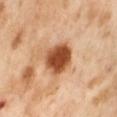workup = total-body-photography surveillance lesion; no biopsy | image = ~15 mm crop, total-body skin-cancer survey | illumination = cross-polarized | anatomic site = the abdomen | lesion diameter = ≈4 mm | patient = female, approximately 55 years of age.